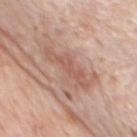Case summary:
– workup: total-body-photography surveillance lesion; no biopsy
– automated metrics: a border-irregularity rating of about 7/10; a nevus-likeness score of about 0/100 and lesion-presence confidence of about 95/100
– anatomic site: the chest
– tile lighting: white-light illumination
– subject: male, aged 78 to 82
– image: total-body-photography crop, ~15 mm field of view